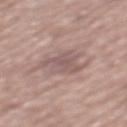{"biopsy_status": "not biopsied; imaged during a skin examination", "patient": {"sex": "male", "age_approx": 75}, "site": "mid back", "image": {"source": "total-body photography crop", "field_of_view_mm": 15}}